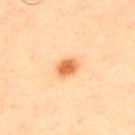patient: male, in their mid-40s
automated metrics: a normalized border contrast of about 9; a border-irregularity rating of about 2/10, a color-variation rating of about 3/10, and peripheral color asymmetry of about 1
tile lighting: cross-polarized illumination
lesion size: ≈2.5 mm
location: the upper back
image source: 15 mm crop, total-body photography